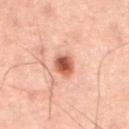Q: How was this image acquired?
A: ~15 mm crop, total-body skin-cancer survey
Q: Patient demographics?
A: male, approximately 60 years of age
Q: Lesion size?
A: about 2.5 mm
Q: What is the anatomic site?
A: the front of the torso
Q: How was the tile lit?
A: cross-polarized